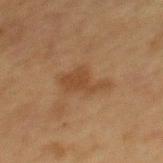workup = total-body-photography surveillance lesion; no biopsy
automated metrics = an average lesion color of about L≈36 a*≈16 b*≈29 (CIELAB) and a normalized border contrast of about 6.5; a nevus-likeness score of about 5/100 and a detector confidence of about 100 out of 100 that the crop contains a lesion
patient = male, about 75 years old
location = the back
diameter = ~4.5 mm (longest diameter)
illumination = cross-polarized
acquisition = ~15 mm tile from a whole-body skin photo